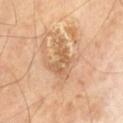follow-up: catalogued during a skin exam; not biopsied | image source: ~15 mm crop, total-body skin-cancer survey | patient: male, aged approximately 70 | location: the left thigh.